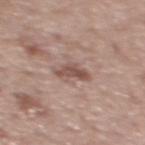| key | value |
|---|---|
| notes | total-body-photography surveillance lesion; no biopsy |
| patient | male, aged around 70 |
| automated metrics | a footprint of about 5 mm², an eccentricity of roughly 0.9, and a shape-asymmetry score of about 0.4 (0 = symmetric); a mean CIELAB color near L≈51 a*≈19 b*≈23, a lesion–skin lightness drop of about 11, and a lesion-to-skin contrast of about 8 (normalized; higher = more distinct); an automated nevus-likeness rating near 0 out of 100 and lesion-presence confidence of about 100/100 |
| lighting | white-light illumination |
| acquisition | 15 mm crop, total-body photography |
| site | the mid back |